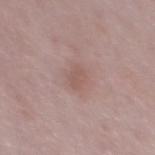workup: imaged on a skin check; not biopsied
diameter: ~2.5 mm (longest diameter)
lighting: white-light
image: total-body-photography crop, ~15 mm field of view
automated metrics: an eccentricity of roughly 0.75 and a symmetry-axis asymmetry near 0.2; a nevus-likeness score of about 0/100 and lesion-presence confidence of about 100/100
patient: male, approximately 50 years of age
anatomic site: the mid back